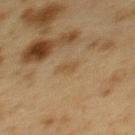  biopsy_status: not biopsied; imaged during a skin examination
  patient:
    sex: female
    age_approx: 40
  lesion_size:
    long_diameter_mm_approx: 2.5
  image:
    source: total-body photography crop
    field_of_view_mm: 15
  automated_metrics:
    area_mm2_approx: 3.0
    eccentricity: 0.85
    shape_asymmetry: 0.4
    lesion_detection_confidence_0_100: 100
  site: upper back
  lighting: cross-polarized